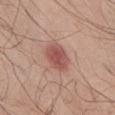Q: Was this lesion biopsied?
A: no biopsy performed (imaged during a skin exam)
Q: What is the lesion's diameter?
A: ≈4 mm
Q: What is the imaging modality?
A: ~15 mm crop, total-body skin-cancer survey
Q: Illumination type?
A: white-light illumination
Q: What are the patient's age and sex?
A: male, aged approximately 50
Q: What is the anatomic site?
A: the chest